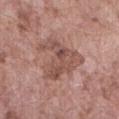Impression: The lesion was photographed on a routine skin check and not biopsied; there is no pathology result. Image and clinical context: Approximately 6 mm at its widest. Cropped from a total-body skin-imaging series; the visible field is about 15 mm. A male subject approximately 75 years of age. The tile uses white-light illumination. The lesion is on the abdomen.